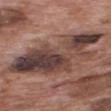- biopsy status — total-body-photography surveillance lesion; no biopsy
- image — ~15 mm crop, total-body skin-cancer survey
- TBP lesion metrics — an area of roughly 39 mm², an outline eccentricity of about 0.85 (0 = round, 1 = elongated), and a shape-asymmetry score of about 0.4 (0 = symmetric); a mean CIELAB color near L≈40 a*≈17 b*≈21, a lesion–skin lightness drop of about 14, and a normalized border contrast of about 11.5
- patient — male, aged 68 to 72
- body site — the back